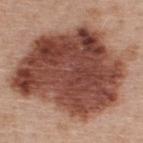Recorded during total-body skin imaging; not selected for excision or biopsy.
Automated image analysis of the tile measured a lesion area of about 90 mm², an eccentricity of roughly 0.65, and a shape-asymmetry score of about 0.25 (0 = symmetric). The analysis additionally found a mean CIELAB color near L≈45 a*≈23 b*≈27 and a lesion–skin lightness drop of about 19. And it measured border irregularity of about 3 on a 0–10 scale, a color-variation rating of about 8/10, and peripheral color asymmetry of about 3.
A male subject, aged 58–62.
Located on the upper back.
A region of skin cropped from a whole-body photographic capture, roughly 15 mm wide.